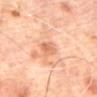Assessment: Captured during whole-body skin photography for melanoma surveillance; the lesion was not biopsied. Image and clinical context: On the back. Imaged with cross-polarized lighting. A close-up tile cropped from a whole-body skin photograph, about 15 mm across. Automated image analysis of the tile measured border irregularity of about 2 on a 0–10 scale and a peripheral color-asymmetry measure near 1. And it measured a classifier nevus-likeness of about 0/100. Approximately 3 mm at its widest. The patient is a male aged 63 to 67.